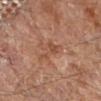biopsy status: no biopsy performed (imaged during a skin exam) | lesion diameter: ~4.5 mm (longest diameter) | lighting: cross-polarized illumination | automated metrics: an area of roughly 4.5 mm², a shape eccentricity near 0.95, and a shape-asymmetry score of about 0.5 (0 = symmetric) | patient: male, aged approximately 85 or older | imaging modality: total-body-photography crop, ~15 mm field of view | body site: the right forearm.